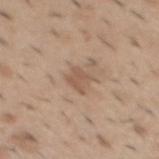follow-up = catalogued during a skin exam; not biopsied | image = ~15 mm crop, total-body skin-cancer survey | subject = male, roughly 40 years of age | location = the mid back.